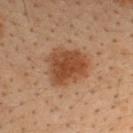Q: Is there a histopathology result?
A: imaged on a skin check; not biopsied
Q: Lesion location?
A: the upper back
Q: Patient demographics?
A: male, about 35 years old
Q: How was this image acquired?
A: ~15 mm tile from a whole-body skin photo
Q: How large is the lesion?
A: ~5 mm (longest diameter)